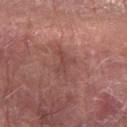Q: Was a biopsy performed?
A: no biopsy performed (imaged during a skin exam)
Q: What are the patient's age and sex?
A: male, aged approximately 65
Q: Where on the body is the lesion?
A: the left forearm
Q: Lesion size?
A: ≈3.5 mm
Q: What kind of image is this?
A: total-body-photography crop, ~15 mm field of view
Q: Illumination type?
A: white-light illumination